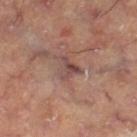* workup — catalogued during a skin exam; not biopsied
* tile lighting — cross-polarized
* automated lesion analysis — a footprint of about 5 mm², an eccentricity of roughly 0.55, and a shape-asymmetry score of about 0.55 (0 = symmetric); a lesion–skin lightness drop of about 9 and a normalized lesion–skin contrast near 7; border irregularity of about 5 on a 0–10 scale, a color-variation rating of about 5.5/10, and radial color variation of about 2
* anatomic site — the right thigh
* size — ~3 mm (longest diameter)
* image — ~15 mm crop, total-body skin-cancer survey